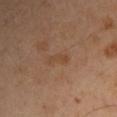Captured during whole-body skin photography for melanoma surveillance; the lesion was not biopsied. The subject is a male approximately 50 years of age. Longest diameter approximately 2.5 mm. Captured under cross-polarized illumination. The lesion is located on the arm. The lesion-visualizer software estimated an average lesion color of about L≈43 a*≈18 b*≈31 (CIELAB), roughly 5 lightness units darker than nearby skin, and a lesion-to-skin contrast of about 5 (normalized; higher = more distinct). And it measured a border-irregularity rating of about 4.5/10, a within-lesion color-variation index near 0/10, and radial color variation of about 0. The software also gave a nevus-likeness score of about 0/100 and lesion-presence confidence of about 100/100. A 15 mm crop from a total-body photograph taken for skin-cancer surveillance.